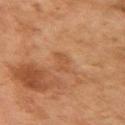The lesion was photographed on a routine skin check and not biopsied; there is no pathology result.
A female patient aged around 60.
A lesion tile, about 15 mm wide, cut from a 3D total-body photograph.
On the left upper arm.
Approximately 2.5 mm at its widest.
Captured under cross-polarized illumination.